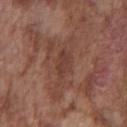Q: How was this image acquired?
A: total-body-photography crop, ~15 mm field of view
Q: What are the patient's age and sex?
A: male, in their mid- to late 70s
Q: What is the anatomic site?
A: the chest